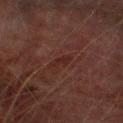Assessment: Recorded during total-body skin imaging; not selected for excision or biopsy. Image and clinical context: A close-up tile cropped from a whole-body skin photograph, about 15 mm across. The lesion is on the left lower leg. A male subject roughly 75 years of age.